The patient is a female approximately 20 years of age; a roughly 15 mm field-of-view crop from a total-body skin photograph; on the head or neck:
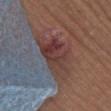* histopathologic diagnosis · a basal cell carcinoma